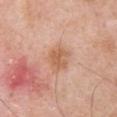Imaged during a routine full-body skin examination; the lesion was not biopsied and no histopathology is available.
From the chest.
The patient is a male in their mid- to late 50s.
A 15 mm close-up tile from a total-body photography series done for melanoma screening.
Imaged with white-light lighting.